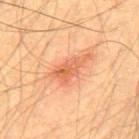Part of a total-body skin-imaging series; this lesion was reviewed on a skin check and was not flagged for biopsy.